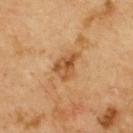| field | value |
|---|---|
| follow-up | no biopsy performed (imaged during a skin exam) |
| lighting | cross-polarized |
| diameter | ~3.5 mm (longest diameter) |
| body site | the upper back |
| image-analysis metrics | a lesion area of about 6 mm² and an outline eccentricity of about 0.7 (0 = round, 1 = elongated); an average lesion color of about L≈50 a*≈21 b*≈39 (CIELAB) and a lesion–skin lightness drop of about 10 |
| imaging modality | ~15 mm tile from a whole-body skin photo |
| subject | male, approximately 70 years of age |